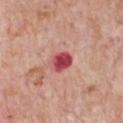Impression: Imaged during a routine full-body skin examination; the lesion was not biopsied and no histopathology is available. Acquisition and patient details: A close-up tile cropped from a whole-body skin photograph, about 15 mm across. This is a white-light tile. Automated tile analysis of the lesion measured an area of roughly 6 mm², an eccentricity of roughly 0.6, and a symmetry-axis asymmetry near 0.2. It also reported a mean CIELAB color near L≈50 a*≈37 b*≈24, roughly 16 lightness units darker than nearby skin, and a lesion-to-skin contrast of about 11 (normalized; higher = more distinct). The analysis additionally found a border-irregularity index near 1.5/10, a color-variation rating of about 4.5/10, and peripheral color asymmetry of about 1.5. And it measured a nevus-likeness score of about 0/100 and a detector confidence of about 100 out of 100 that the crop contains a lesion. About 3 mm across. A male subject roughly 60 years of age. The lesion is on the chest.